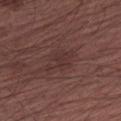The lesion was tiled from a total-body skin photograph and was not biopsied. A lesion tile, about 15 mm wide, cut from a 3D total-body photograph. The patient is a male in their mid- to late 60s. Approximately 4 mm at its widest. The tile uses white-light illumination. The lesion is on the left upper arm.